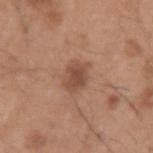No biopsy was performed on this lesion — it was imaged during a full skin examination and was not determined to be concerning.
A male patient, aged 48–52.
From the left upper arm.
A region of skin cropped from a whole-body photographic capture, roughly 15 mm wide.
The lesion's longest dimension is about 3 mm.
Imaged with white-light lighting.
Automated image analysis of the tile measured an area of roughly 7 mm², an outline eccentricity of about 0.6 (0 = round, 1 = elongated), and a shape-asymmetry score of about 0.2 (0 = symmetric).